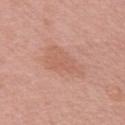Q: How was this image acquired?
A: total-body-photography crop, ~15 mm field of view
Q: What are the patient's age and sex?
A: female, roughly 50 years of age
Q: What is the anatomic site?
A: the right upper arm